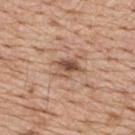A male subject, roughly 70 years of age.
The lesion's longest dimension is about 3 mm.
On the upper back.
A roughly 15 mm field-of-view crop from a total-body skin photograph.
The tile uses white-light illumination.
The total-body-photography lesion software estimated a nevus-likeness score of about 65/100.